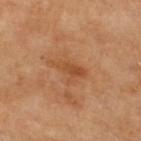  biopsy_status: not biopsied; imaged during a skin examination
  image:
    source: total-body photography crop
    field_of_view_mm: 15
  lesion_size:
    long_diameter_mm_approx: 4.0
  automated_metrics:
    eccentricity: 0.85
    shape_asymmetry: 0.45
    vs_skin_contrast_norm: 6.0
    border_irregularity_0_10: 5.0
    color_variation_0_10: 3.5
    peripheral_color_asymmetry: 1.0
    nevus_likeness_0_100: 0
    lesion_detection_confidence_0_100: 100
  patient:
    sex: female
    age_approx: 60
  lighting: cross-polarized
  site: left lower leg